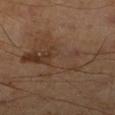Impression:
This lesion was catalogued during total-body skin photography and was not selected for biopsy.
Background:
Imaged with cross-polarized lighting. A male subject aged around 45. The lesion is located on the right lower leg. Longest diameter approximately 10 mm. A 15 mm crop from a total-body photograph taken for skin-cancer surveillance.